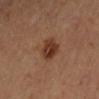biopsy status — no biopsy performed (imaged during a skin exam)
automated metrics — a mean CIELAB color near L≈34 a*≈21 b*≈28 and a lesion–skin lightness drop of about 10; lesion-presence confidence of about 100/100
body site — the left forearm
size — about 3 mm
image source — ~15 mm tile from a whole-body skin photo
subject — female, aged 43–47
lighting — cross-polarized